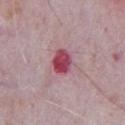Q: Was this lesion biopsied?
A: catalogued during a skin exam; not biopsied
Q: What is the imaging modality?
A: ~15 mm crop, total-body skin-cancer survey
Q: What is the anatomic site?
A: the chest
Q: Patient demographics?
A: male, aged 63 to 67
Q: Illumination type?
A: white-light illumination
Q: How large is the lesion?
A: ~3 mm (longest diameter)